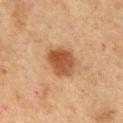workup: catalogued during a skin exam; not biopsied
lesion diameter: ~4 mm (longest diameter)
location: the chest
subject: male, about 75 years old
image source: ~15 mm crop, total-body skin-cancer survey